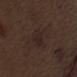Acquisition and patient details: The lesion is on the left upper arm. The tile uses white-light illumination. A male subject roughly 70 years of age. A 15 mm close-up extracted from a 3D total-body photography capture. The total-body-photography lesion software estimated a footprint of about 6.5 mm² and a symmetry-axis asymmetry near 0.2. The software also gave a mean CIELAB color near L≈22 a*≈13 b*≈16 and a normalized border contrast of about 6. It also reported a within-lesion color-variation index near 2/10 and radial color variation of about 0.5.